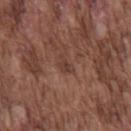Image and clinical context: A male patient, roughly 75 years of age. The lesion is located on the chest. A roughly 15 mm field-of-view crop from a total-body skin photograph.